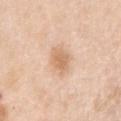<case>
  <image>
    <source>total-body photography crop</source>
    <field_of_view_mm>15</field_of_view_mm>
  </image>
  <lesion_size>
    <long_diameter_mm_approx>3.5</long_diameter_mm_approx>
  </lesion_size>
  <automated_metrics>
    <area_mm2_approx>7.0</area_mm2_approx>
    <eccentricity>0.75</eccentricity>
    <nevus_likeness_0_100>50</nevus_likeness_0_100>
    <lesion_detection_confidence_0_100>100</lesion_detection_confidence_0_100>
  </automated_metrics>
  <patient>
    <sex>female</sex>
    <age_approx>45</age_approx>
  </patient>
  <site>arm</site>
</case>